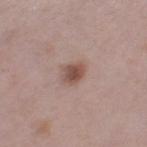  lighting: white-light
  patient:
    sex: female
    age_approx: 40
  image:
    source: total-body photography crop
    field_of_view_mm: 15
  site: left thigh